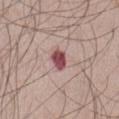follow-up: catalogued during a skin exam; not biopsied | location: the abdomen | lesion diameter: ≈3 mm | lighting: white-light | image source: 15 mm crop, total-body photography | TBP lesion metrics: an area of roughly 4.5 mm², an eccentricity of roughly 0.7, and a shape-asymmetry score of about 0.25 (0 = symmetric); a lesion color around L≈49 a*≈27 b*≈18 in CIELAB and a normalized lesion–skin contrast near 11.5; a nevus-likeness score of about 0/100 and lesion-presence confidence of about 100/100 | subject: male, about 50 years old.